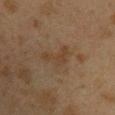workup = total-body-photography surveillance lesion; no biopsy
acquisition = ~15 mm crop, total-body skin-cancer survey
patient = female, in their 40s
lighting = cross-polarized illumination
anatomic site = the arm
lesion diameter = ~3.5 mm (longest diameter)